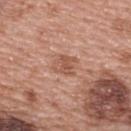Impression: Captured during whole-body skin photography for melanoma surveillance; the lesion was not biopsied. Image and clinical context: A close-up tile cropped from a whole-body skin photograph, about 15 mm across. An algorithmic analysis of the crop reported a lesion-to-skin contrast of about 6 (normalized; higher = more distinct). The analysis additionally found a border-irregularity rating of about 3/10, a color-variation rating of about 2/10, and a peripheral color-asymmetry measure near 1. A female subject, aged 58 to 62. This is a white-light tile. Measured at roughly 2.5 mm in maximum diameter. On the upper back.